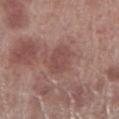Background:
Located on the right lower leg. About 3.5 mm across. The subject is a male aged 68 to 72. The tile uses white-light illumination. This image is a 15 mm lesion crop taken from a total-body photograph.